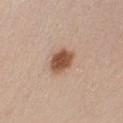follow-up = catalogued during a skin exam; not biopsied
subject = female, aged 23 to 27
anatomic site = the front of the torso
lighting = white-light
image source = 15 mm crop, total-body photography
diameter = about 3.5 mm